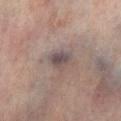Recorded during total-body skin imaging; not selected for excision or biopsy.